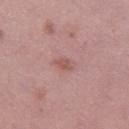Impression:
This lesion was catalogued during total-body skin photography and was not selected for biopsy.
Acquisition and patient details:
This is a white-light tile. The recorded lesion diameter is about 2.5 mm. The lesion is on the left thigh. A female patient aged around 50. A close-up tile cropped from a whole-body skin photograph, about 15 mm across.